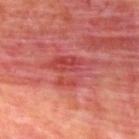The lesion was photographed on a routine skin check and not biopsied; there is no pathology result. The lesion is on the upper back. Cropped from a whole-body photographic skin survey; the tile spans about 15 mm. A male subject, aged 68–72. The lesion's longest dimension is about 6.5 mm. An algorithmic analysis of the crop reported an outline eccentricity of about 0.75 (0 = round, 1 = elongated). And it measured roughly 9 lightness units darker than nearby skin and a normalized border contrast of about 6. The software also gave a border-irregularity index near 5.5/10 and a within-lesion color-variation index near 7/10. The software also gave a nevus-likeness score of about 0/100 and lesion-presence confidence of about 90/100. Imaged with cross-polarized lighting.